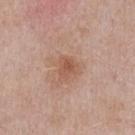Assessment:
Recorded during total-body skin imaging; not selected for excision or biopsy.
Acquisition and patient details:
Approximately 2.5 mm at its widest. A 15 mm close-up extracted from a 3D total-body photography capture. The lesion is on the chest. A male subject, aged 53 to 57.